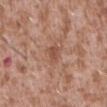Context: A male patient, roughly 45 years of age. This is a white-light tile. The lesion-visualizer software estimated a footprint of about 4 mm² and a shape eccentricity near 0.7. And it measured internal color variation of about 2 on a 0–10 scale. The analysis additionally found a classifier nevus-likeness of about 0/100 and lesion-presence confidence of about 100/100. From the abdomen. This image is a 15 mm lesion crop taken from a total-body photograph.